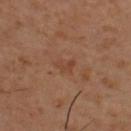biopsy status=catalogued during a skin exam; not biopsied
site=the upper back
lighting=cross-polarized
subject=male, in their mid- to late 50s
image=15 mm crop, total-body photography
lesion diameter=≈2.5 mm
image-analysis metrics=an area of roughly 3 mm², an eccentricity of roughly 0.75, and a symmetry-axis asymmetry near 0.45; about 5 CIELAB-L* units darker than the surrounding skin and a normalized border contrast of about 5; a border-irregularity rating of about 4/10, a within-lesion color-variation index near 1/10, and a peripheral color-asymmetry measure near 0.5